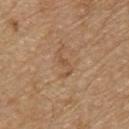The lesion was tiled from a total-body skin photograph and was not biopsied.
The lesion's longest dimension is about 3.5 mm.
A 15 mm close-up extracted from a 3D total-body photography capture.
Captured under white-light illumination.
On the chest.
The subject is a male about 70 years old.